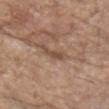<tbp_lesion>
  <biopsy_status>not biopsied; imaged during a skin examination</biopsy_status>
  <automated_metrics>
    <area_mm2_approx>2.5</area_mm2_approx>
    <eccentricity>0.95</eccentricity>
    <shape_asymmetry>0.25</shape_asymmetry>
    <cielab_L>48</cielab_L>
    <cielab_a>18</cielab_a>
    <cielab_b>27</cielab_b>
    <vs_skin_darker_L>9.0</vs_skin_darker_L>
    <vs_skin_contrast_norm>7.0</vs_skin_contrast_norm>
    <border_irregularity_0_10>3.5</border_irregularity_0_10>
    <color_variation_0_10>0.0</color_variation_0_10>
    <peripheral_color_asymmetry>0.0</peripheral_color_asymmetry>
  </automated_metrics>
  <patient>
    <sex>male</sex>
    <age_approx>85+</age_approx>
  </patient>
  <lighting>white-light</lighting>
  <image>
    <source>total-body photography crop</source>
    <field_of_view_mm>15</field_of_view_mm>
  </image>
  <site>chest</site>
  <lesion_size>
    <long_diameter_mm_approx>3.0</long_diameter_mm_approx>
  </lesion_size>
</tbp_lesion>